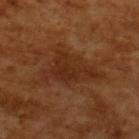Findings:
• tile lighting · cross-polarized illumination
• imaging modality · ~15 mm tile from a whole-body skin photo
• diameter · ≈6.5 mm
• subject · male, approximately 65 years of age